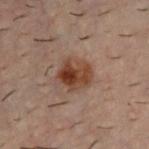  biopsy_status: not biopsied; imaged during a skin examination
  image:
    source: total-body photography crop
    field_of_view_mm: 15
  lighting: cross-polarized
  patient:
    sex: male
    age_approx: 30
  site: chest
  automated_metrics:
    area_mm2_approx: 10.0
    eccentricity: 0.45
    cielab_L: 33
    cielab_a: 17
    cielab_b: 24
    vs_skin_darker_L: 9.0
    vs_skin_contrast_norm: 9.5
    nevus_likeness_0_100: 100
    lesion_detection_confidence_0_100: 100